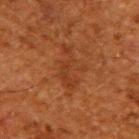A male subject in their 60s.
A close-up tile cropped from a whole-body skin photograph, about 15 mm across.
On the upper back.
Automated tile analysis of the lesion measured a lesion area of about 11 mm², a shape eccentricity near 0.9, and a shape-asymmetry score of about 0.35 (0 = symmetric). And it measured an average lesion color of about L≈30 a*≈21 b*≈30 (CIELAB), a lesion–skin lightness drop of about 5, and a lesion-to-skin contrast of about 5 (normalized; higher = more distinct). It also reported a detector confidence of about 100 out of 100 that the crop contains a lesion.
This is a cross-polarized tile.
Measured at roughly 6 mm in maximum diameter.